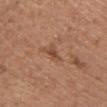This lesion was catalogued during total-body skin photography and was not selected for biopsy.
From the front of the torso.
Automated tile analysis of the lesion measured a symmetry-axis asymmetry near 0.35. The software also gave a mean CIELAB color near L≈48 a*≈22 b*≈31 and a lesion-to-skin contrast of about 6 (normalized; higher = more distinct). It also reported a border-irregularity index near 3.5/10, internal color variation of about 1 on a 0–10 scale, and peripheral color asymmetry of about 0. And it measured a classifier nevus-likeness of about 0/100 and lesion-presence confidence of about 95/100.
A female subject, aged approximately 55.
A lesion tile, about 15 mm wide, cut from a 3D total-body photograph.
The lesion's longest dimension is about 2.5 mm.
Captured under white-light illumination.